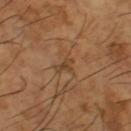Imaged during a routine full-body skin examination; the lesion was not biopsied and no histopathology is available. A male patient approximately 65 years of age. A 15 mm close-up tile from a total-body photography series done for melanoma screening. Longest diameter approximately 2.5 mm. The lesion is on the left thigh. Captured under cross-polarized illumination.